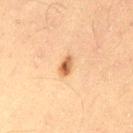Q: Is there a histopathology result?
A: imaged on a skin check; not biopsied
Q: Illumination type?
A: cross-polarized
Q: What did automated image analysis measure?
A: border irregularity of about 2.5 on a 0–10 scale, internal color variation of about 3 on a 0–10 scale, and a peripheral color-asymmetry measure near 1
Q: Who is the patient?
A: male, aged 63–67
Q: How was this image acquired?
A: total-body-photography crop, ~15 mm field of view
Q: Lesion size?
A: ~2.5 mm (longest diameter)
Q: Where on the body is the lesion?
A: the left thigh